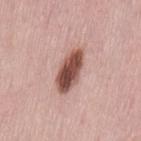The lesion was photographed on a routine skin check and not biopsied; there is no pathology result.
A female subject, approximately 50 years of age.
The lesion is on the lower back.
A lesion tile, about 15 mm wide, cut from a 3D total-body photograph.
The lesion-visualizer software estimated border irregularity of about 2 on a 0–10 scale and internal color variation of about 6 on a 0–10 scale.
Captured under white-light illumination.
The lesion's longest dimension is about 5.5 mm.